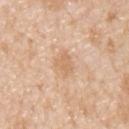biopsy status: no biopsy performed (imaged during a skin exam); body site: the upper back; diameter: ≈3 mm; tile lighting: white-light; imaging modality: total-body-photography crop, ~15 mm field of view; patient: male, aged 43–47.